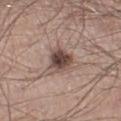  biopsy_status: not biopsied; imaged during a skin examination
  image:
    source: total-body photography crop
    field_of_view_mm: 15
  automated_metrics:
    area_mm2_approx: 7.0
    eccentricity: 0.45
    cielab_L: 45
    cielab_a: 17
    cielab_b: 21
    vs_skin_darker_L: 15.0
    vs_skin_contrast_norm: 11.0
    color_variation_0_10: 5.0
    peripheral_color_asymmetry: 1.5
  lesion_size:
    long_diameter_mm_approx: 3.5
  lighting: white-light
  site: left lower leg
  patient:
    sex: male
    age_approx: 40